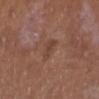| feature | finding |
|---|---|
| notes | catalogued during a skin exam; not biopsied |
| subject | male, roughly 65 years of age |
| TBP lesion metrics | about 6 CIELAB-L* units darker than the surrounding skin and a lesion-to-skin contrast of about 5.5 (normalized; higher = more distinct); a nevus-likeness score of about 0/100 and lesion-presence confidence of about 100/100 |
| diameter | about 3 mm |
| imaging modality | total-body-photography crop, ~15 mm field of view |
| anatomic site | the leg |
| lighting | white-light |